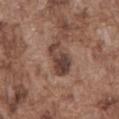Findings:
- follow-up: total-body-photography surveillance lesion; no biopsy
- anatomic site: the abdomen
- imaging modality: ~15 mm crop, total-body skin-cancer survey
- illumination: white-light illumination
- subject: male, aged 73 to 77